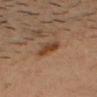No biopsy was performed on this lesion — it was imaged during a full skin examination and was not determined to be concerning.
Cropped from a whole-body photographic skin survey; the tile spans about 15 mm.
A male subject in their mid- to late 30s.
Located on the head or neck.
This is a cross-polarized tile.
About 3 mm across.